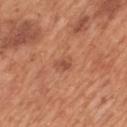Part of a total-body skin-imaging series; this lesion was reviewed on a skin check and was not flagged for biopsy. Located on the back. The recorded lesion diameter is about 2.5 mm. A 15 mm close-up extracted from a 3D total-body photography capture. The lesion-visualizer software estimated a classifier nevus-likeness of about 10/100. A male patient aged 63 to 67.